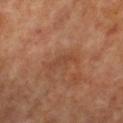Part of a total-body skin-imaging series; this lesion was reviewed on a skin check and was not flagged for biopsy.